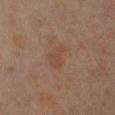{"biopsy_status": "not biopsied; imaged during a skin examination", "site": "left lower leg", "image": {"source": "total-body photography crop", "field_of_view_mm": 15}, "lighting": "cross-polarized", "patient": {"sex": "female", "age_approx": 55}, "lesion_size": {"long_diameter_mm_approx": 3.5}}